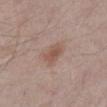No biopsy was performed on this lesion — it was imaged during a full skin examination and was not determined to be concerning.
Imaged with white-light lighting.
A male patient, roughly 55 years of age.
The lesion is located on the abdomen.
A close-up tile cropped from a whole-body skin photograph, about 15 mm across.
The recorded lesion diameter is about 2.5 mm.